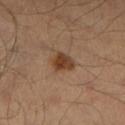notes: catalogued during a skin exam; not biopsied
automated lesion analysis: a border-irregularity index near 2/10 and radial color variation of about 1
tile lighting: cross-polarized illumination
anatomic site: the right lower leg
patient: male, approximately 40 years of age
size: ~3 mm (longest diameter)
image source: 15 mm crop, total-body photography